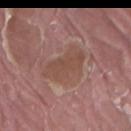From the right thigh. This image is a 15 mm lesion crop taken from a total-body photograph. Approximately 6 mm at its widest. Captured under white-light illumination. A male patient, aged around 40.